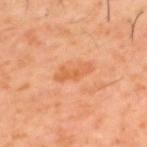Imaged during a routine full-body skin examination; the lesion was not biopsied and no histopathology is available.
Located on the mid back.
Measured at roughly 4 mm in maximum diameter.
A male subject, aged 58 to 62.
The total-body-photography lesion software estimated a footprint of about 5 mm², an eccentricity of roughly 0.95, and two-axis asymmetry of about 0.4. The analysis additionally found a border-irregularity rating of about 5/10 and a within-lesion color-variation index near 0.5/10.
This is a cross-polarized tile.
A 15 mm close-up tile from a total-body photography series done for melanoma screening.